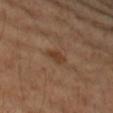biopsy_status: not biopsied; imaged during a skin examination
image:
  source: total-body photography crop
  field_of_view_mm: 15
lesion_size:
  long_diameter_mm_approx: 3.0
site: right forearm
patient:
  sex: female
  age_approx: 60
lighting: cross-polarized